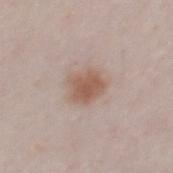Q: Was this lesion biopsied?
A: no biopsy performed (imaged during a skin exam)
Q: How was this image acquired?
A: 15 mm crop, total-body photography
Q: Patient demographics?
A: male, aged 23 to 27
Q: Lesion location?
A: the mid back
Q: What lighting was used for the tile?
A: white-light
Q: What is the lesion's diameter?
A: about 3.5 mm